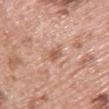Captured during whole-body skin photography for melanoma surveillance; the lesion was not biopsied.
Cropped from a whole-body photographic skin survey; the tile spans about 15 mm.
On the chest.
The subject is a female aged 58 to 62.